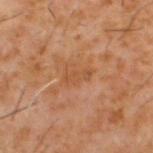The lesion was photographed on a routine skin check and not biopsied; there is no pathology result.
A 15 mm crop from a total-body photograph taken for skin-cancer surveillance.
On the upper back.
Automated tile analysis of the lesion measured a nevus-likeness score of about 0/100 and a lesion-detection confidence of about 100/100.
Imaged with cross-polarized lighting.
A male subject about 60 years old.